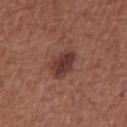This lesion was catalogued during total-body skin photography and was not selected for biopsy.
The patient is a male aged approximately 65.
Located on the abdomen.
Imaged with white-light lighting.
A 15 mm crop from a total-body photograph taken for skin-cancer surveillance.
Longest diameter approximately 3.5 mm.
An algorithmic analysis of the crop reported an area of roughly 7 mm², a shape eccentricity near 0.65, and a shape-asymmetry score of about 0.2 (0 = symmetric). The analysis additionally found a classifier nevus-likeness of about 80/100 and lesion-presence confidence of about 100/100.